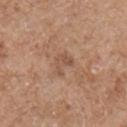  biopsy_status: not biopsied; imaged during a skin examination
  site: chest
  patient:
    sex: male
    age_approx: 55
  image:
    source: total-body photography crop
    field_of_view_mm: 15
  automated_metrics:
    border_irregularity_0_10: 5.5
    color_variation_0_10: 2.0
    peripheral_color_asymmetry: 1.0
    nevus_likeness_0_100: 0
  lighting: white-light
  lesion_size:
    long_diameter_mm_approx: 3.0